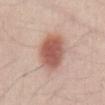notes = catalogued during a skin exam; not biopsied
anatomic site = the abdomen
subject = male, in their mid- to late 20s
size = about 5 mm
lighting = white-light
imaging modality = ~15 mm crop, total-body skin-cancer survey